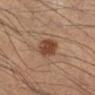The lesion was tiled from a total-body skin photograph and was not biopsied. A male patient roughly 40 years of age. On the left lower leg. Automated tile analysis of the lesion measured a footprint of about 7 mm² and a shape eccentricity near 0.75. The software also gave a border-irregularity rating of about 1.5/10 and radial color variation of about 1. The software also gave a classifier nevus-likeness of about 95/100 and a detector confidence of about 100 out of 100 that the crop contains a lesion. The tile uses cross-polarized illumination. Longest diameter approximately 4 mm. Cropped from a total-body skin-imaging series; the visible field is about 15 mm.